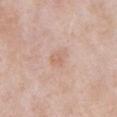Clinical impression:
No biopsy was performed on this lesion — it was imaged during a full skin examination and was not determined to be concerning.
Clinical summary:
Cropped from a total-body skin-imaging series; the visible field is about 15 mm. Located on the abdomen. The recorded lesion diameter is about 2.5 mm. The tile uses white-light illumination. A male subject, aged around 55. An algorithmic analysis of the crop reported a footprint of about 4 mm² and a symmetry-axis asymmetry near 0.2. The software also gave a mean CIELAB color near L≈65 a*≈19 b*≈29, roughly 6 lightness units darker than nearby skin, and a normalized border contrast of about 4.5. The analysis additionally found border irregularity of about 2 on a 0–10 scale, a within-lesion color-variation index near 2/10, and radial color variation of about 0.5. The software also gave an automated nevus-likeness rating near 0 out of 100 and a lesion-detection confidence of about 100/100.